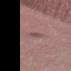Impression:
This lesion was catalogued during total-body skin photography and was not selected for biopsy.
Context:
Captured under white-light illumination. A lesion tile, about 15 mm wide, cut from a 3D total-body photograph. A female subject, about 50 years old. An algorithmic analysis of the crop reported a border-irregularity index near 5/10, internal color variation of about 0 on a 0–10 scale, and peripheral color asymmetry of about 0. The analysis additionally found an automated nevus-likeness rating near 0 out of 100 and a detector confidence of about 90 out of 100 that the crop contains a lesion. The lesion is located on the left thigh. Longest diameter approximately 2.5 mm.